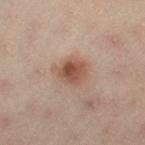Q: Is there a histopathology result?
A: total-body-photography surveillance lesion; no biopsy
Q: Lesion location?
A: the right thigh
Q: What did automated image analysis measure?
A: an average lesion color of about L≈53 a*≈21 b*≈28 (CIELAB), about 12 CIELAB-L* units darker than the surrounding skin, and a lesion-to-skin contrast of about 8.5 (normalized; higher = more distinct)
Q: What is the imaging modality?
A: total-body-photography crop, ~15 mm field of view
Q: How large is the lesion?
A: ≈3.5 mm
Q: What lighting was used for the tile?
A: cross-polarized
Q: Who is the patient?
A: female, aged around 55